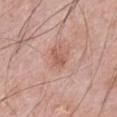Assessment:
Part of a total-body skin-imaging series; this lesion was reviewed on a skin check and was not flagged for biopsy.
Image and clinical context:
A region of skin cropped from a whole-body photographic capture, roughly 15 mm wide. On the arm. Imaged with white-light lighting. A male patient, aged 63–67. Approximately 2.5 mm at its widest.